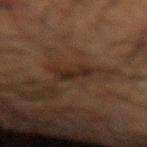<tbp_lesion>
  <biopsy_status>not biopsied; imaged during a skin examination</biopsy_status>
  <lighting>cross-polarized</lighting>
  <automated_metrics>
    <cielab_L>19</cielab_L>
    <cielab_a>12</cielab_a>
    <cielab_b>19</cielab_b>
    <vs_skin_darker_L>6.0</vs_skin_darker_L>
    <vs_skin_contrast_norm>8.0</vs_skin_contrast_norm>
  </automated_metrics>
  <image>
    <source>total-body photography crop</source>
    <field_of_view_mm>15</field_of_view_mm>
  </image>
  <site>left forearm</site>
  <patient>
    <sex>male</sex>
    <age_approx>60</age_approx>
  </patient>
</tbp_lesion>